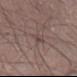{
  "automated_metrics": {
    "area_mm2_approx": 2.5,
    "eccentricity": 0.9,
    "shape_asymmetry": 0.25,
    "cielab_L": 44,
    "cielab_a": 15,
    "cielab_b": 18,
    "vs_skin_darker_L": 6.0,
    "vs_skin_contrast_norm": 5.0
  },
  "lesion_size": {
    "long_diameter_mm_approx": 2.5
  },
  "image": {
    "source": "total-body photography crop",
    "field_of_view_mm": 15
  },
  "patient": {
    "sex": "male",
    "age_approx": 65
  },
  "site": "left thigh"
}